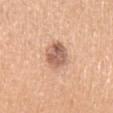  biopsy_status: not biopsied; imaged during a skin examination
  patient:
    sex: female
    age_approx: 65
  lighting: white-light
  site: left upper arm
  image:
    source: total-body photography crop
    field_of_view_mm: 15
  automated_metrics:
    eccentricity: 0.65
    shape_asymmetry: 0.15
    cielab_L: 61
    cielab_a: 21
    cielab_b: 30
    vs_skin_darker_L: 13.0
    vs_skin_contrast_norm: 8.0
    border_irregularity_0_10: 1.5
    color_variation_0_10: 5.0
    peripheral_color_asymmetry: 2.0
  lesion_size:
    long_diameter_mm_approx: 4.0